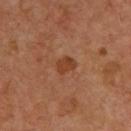Q: Was a biopsy performed?
A: total-body-photography surveillance lesion; no biopsy
Q: How was this image acquired?
A: total-body-photography crop, ~15 mm field of view
Q: What is the lesion's diameter?
A: about 2 mm
Q: Who is the patient?
A: male, in their mid- to late 60s
Q: Where on the body is the lesion?
A: the upper back
Q: Illumination type?
A: cross-polarized illumination